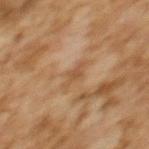Impression: The lesion was photographed on a routine skin check and not biopsied; there is no pathology result. Background: The subject is a female aged approximately 60. Automated tile analysis of the lesion measured an area of roughly 3.5 mm², an eccentricity of roughly 0.85, and a shape-asymmetry score of about 0.4 (0 = symmetric). From the upper back. A 15 mm close-up tile from a total-body photography series done for melanoma screening. The tile uses cross-polarized illumination.